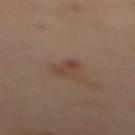Part of a total-body skin-imaging series; this lesion was reviewed on a skin check and was not flagged for biopsy. A region of skin cropped from a whole-body photographic capture, roughly 15 mm wide. A female subject, aged around 55. The lesion's longest dimension is about 2.5 mm. The tile uses cross-polarized illumination. The lesion is located on the upper back.